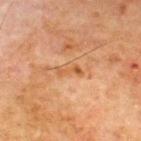{
  "biopsy_status": "not biopsied; imaged during a skin examination",
  "image": {
    "source": "total-body photography crop",
    "field_of_view_mm": 15
  },
  "lesion_size": {
    "long_diameter_mm_approx": 3.0
  },
  "patient": {
    "sex": "male",
    "age_approx": 70
  },
  "site": "upper back"
}